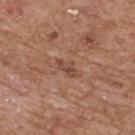Assessment: This lesion was catalogued during total-body skin photography and was not selected for biopsy. Context: A male subject aged 63–67. On the upper back. A region of skin cropped from a whole-body photographic capture, roughly 15 mm wide.